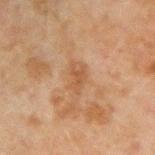Impression:
Part of a total-body skin-imaging series; this lesion was reviewed on a skin check and was not flagged for biopsy.
Background:
The lesion is located on the right forearm. About 3 mm across. A male subject, about 45 years old. A 15 mm close-up tile from a total-body photography series done for melanoma screening. The lesion-visualizer software estimated a within-lesion color-variation index near 3/10 and peripheral color asymmetry of about 1. The software also gave an automated nevus-likeness rating near 0 out of 100 and lesion-presence confidence of about 100/100. Imaged with cross-polarized lighting.